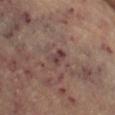From the left thigh.
A 15 mm close-up tile from a total-body photography series done for melanoma screening.
The lesion-visualizer software estimated a border-irregularity index near 3.5/10 and a within-lesion color-variation index near 2.5/10.
About 3 mm across.
A female subject in their mid- to late 60s.
This is a cross-polarized tile.